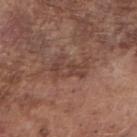biopsy status: no biopsy performed (imaged during a skin exam)
diameter: ≈4.5 mm
location: the right forearm
image source: total-body-photography crop, ~15 mm field of view
patient: male, in their mid-70s
automated metrics: a lesion area of about 8.5 mm², a shape eccentricity near 0.85, and a symmetry-axis asymmetry near 0.45; a mean CIELAB color near L≈42 a*≈20 b*≈24, roughly 7 lightness units darker than nearby skin, and a lesion-to-skin contrast of about 6 (normalized; higher = more distinct); a color-variation rating of about 3.5/10 and a peripheral color-asymmetry measure near 1.5
illumination: white-light illumination